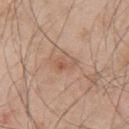{
  "biopsy_status": "not biopsied; imaged during a skin examination",
  "automated_metrics": {
    "area_mm2_approx": 3.0,
    "eccentricity": 0.9,
    "shape_asymmetry": 0.5,
    "border_irregularity_0_10": 5.5,
    "color_variation_0_10": 2.0,
    "peripheral_color_asymmetry": 0.5,
    "nevus_likeness_0_100": 0,
    "lesion_detection_confidence_0_100": 100
  },
  "lighting": "white-light",
  "patient": {
    "sex": "male",
    "age_approx": 55
  },
  "site": "upper back",
  "lesion_size": {
    "long_diameter_mm_approx": 3.0
  },
  "image": {
    "source": "total-body photography crop",
    "field_of_view_mm": 15
  }
}